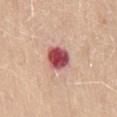Imaged during a routine full-body skin examination; the lesion was not biopsied and no histopathology is available. A female patient, aged around 55. A 15 mm crop from a total-body photograph taken for skin-cancer surveillance. The lesion is on the back.